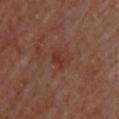Captured during whole-body skin photography for melanoma surveillance; the lesion was not biopsied. The lesion's longest dimension is about 2.5 mm. The lesion is on the upper back. Automated tile analysis of the lesion measured a nevus-likeness score of about 0/100 and a detector confidence of about 100 out of 100 that the crop contains a lesion. Cropped from a whole-body photographic skin survey; the tile spans about 15 mm. A female subject, aged approximately 60. The tile uses cross-polarized illumination.